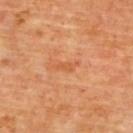{
  "biopsy_status": "not biopsied; imaged during a skin examination",
  "lesion_size": {
    "long_diameter_mm_approx": 3.0
  },
  "automated_metrics": {
    "cielab_L": 56,
    "cielab_a": 28,
    "cielab_b": 41,
    "vs_skin_darker_L": 6.0,
    "vs_skin_contrast_norm": 5.0,
    "border_irregularity_0_10": 4.5,
    "color_variation_0_10": 0.0,
    "peripheral_color_asymmetry": 0.0,
    "nevus_likeness_0_100": 0,
    "lesion_detection_confidence_0_100": 100
  },
  "image": {
    "source": "total-body photography crop",
    "field_of_view_mm": 15
  },
  "patient": {
    "sex": "male",
    "age_approx": 60
  },
  "lighting": "cross-polarized",
  "site": "upper back"
}